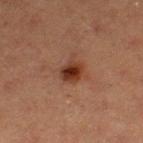The lesion was tiled from a total-body skin photograph and was not biopsied.
Located on the leg.
A female subject, about 40 years old.
The total-body-photography lesion software estimated two-axis asymmetry of about 0.2. The software also gave a mean CIELAB color near L≈28 a*≈21 b*≈26, about 11 CIELAB-L* units darker than the surrounding skin, and a normalized lesion–skin contrast near 11.
A 15 mm crop from a total-body photograph taken for skin-cancer surveillance.